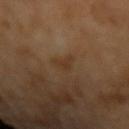notes: no biopsy performed (imaged during a skin exam) | automated lesion analysis: a footprint of about 3 mm², an outline eccentricity of about 0.7 (0 = round, 1 = elongated), and two-axis asymmetry of about 0.5; a mean CIELAB color near L≈33 a*≈16 b*≈29, a lesion–skin lightness drop of about 5, and a normalized border contrast of about 5.5 | acquisition: total-body-photography crop, ~15 mm field of view | patient: male, aged 63–67.